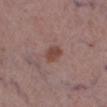Clinical impression: Recorded during total-body skin imaging; not selected for excision or biopsy. Clinical summary: A female subject aged approximately 65. Longest diameter approximately 3 mm. The lesion is located on the leg. A 15 mm close-up tile from a total-body photography series done for melanoma screening.